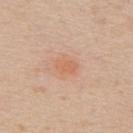<lesion>
<biopsy_status>not biopsied; imaged during a skin examination</biopsy_status>
<patient>
  <sex>male</sex>
  <age_approx>60</age_approx>
</patient>
<site>upper back</site>
<image>
  <source>total-body photography crop</source>
  <field_of_view_mm>15</field_of_view_mm>
</image>
<lighting>white-light</lighting>
</lesion>